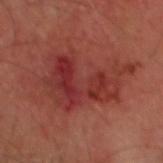Diagnosis: Histopathological examination showed a squamous cell carcinoma in situ (malignant).